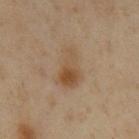Impression: Part of a total-body skin-imaging series; this lesion was reviewed on a skin check and was not flagged for biopsy. Clinical summary: Measured at roughly 5 mm in maximum diameter. Captured under cross-polarized illumination. The patient is a male about 60 years old. A 15 mm close-up tile from a total-body photography series done for melanoma screening. Located on the chest.